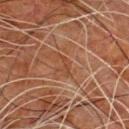| feature | finding |
|---|---|
| body site | the chest |
| illumination | cross-polarized |
| imaging modality | ~15 mm tile from a whole-body skin photo |
| size | about 3 mm |
| patient | male, approximately 80 years of age |